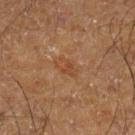follow-up=catalogued during a skin exam; not biopsied
lesion size=~3 mm (longest diameter)
image-analysis metrics=a lesion color around L≈38 a*≈18 b*≈29 in CIELAB, a lesion–skin lightness drop of about 5, and a normalized lesion–skin contrast near 4.5; a lesion-detection confidence of about 100/100
patient=male, about 65 years old
site=the right lower leg
image source=total-body-photography crop, ~15 mm field of view
lighting=cross-polarized